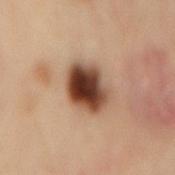Impression:
The lesion was tiled from a total-body skin photograph and was not biopsied.
Image and clinical context:
A female patient roughly 60 years of age. Located on the back. The total-body-photography lesion software estimated a footprint of about 15 mm² and an outline eccentricity of about 0.65 (0 = round, 1 = elongated). The analysis additionally found an average lesion color of about L≈43 a*≈21 b*≈29 (CIELAB), about 21 CIELAB-L* units darker than the surrounding skin, and a normalized lesion–skin contrast near 14.5. Cropped from a total-body skin-imaging series; the visible field is about 15 mm. Imaged with cross-polarized lighting.